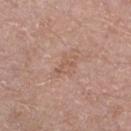  biopsy_status: not biopsied; imaged during a skin examination
  lesion_size:
    long_diameter_mm_approx: 3.5
  patient:
    sex: female
    age_approx: 50
  automated_metrics:
    vs_skin_darker_L: 6.0
    lesion_detection_confidence_0_100: 100
  image:
    source: total-body photography crop
    field_of_view_mm: 15
  lighting: white-light
  site: right upper arm